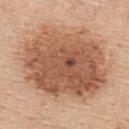| feature | finding |
|---|---|
| notes | imaged on a skin check; not biopsied |
| body site | the upper back |
| subject | female, aged 43 to 47 |
| imaging modality | ~15 mm tile from a whole-body skin photo |